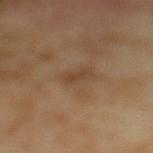Impression:
Recorded during total-body skin imaging; not selected for excision or biopsy.